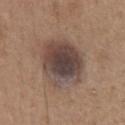This lesion was catalogued during total-body skin photography and was not selected for biopsy. A 15 mm close-up tile from a total-body photography series done for melanoma screening. Measured at roughly 6 mm in maximum diameter. The lesion is located on the chest. The tile uses white-light illumination. An algorithmic analysis of the crop reported about 13 CIELAB-L* units darker than the surrounding skin and a normalized border contrast of about 11. And it measured an automated nevus-likeness rating near 30 out of 100. A male patient aged around 65.